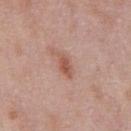| key | value |
|---|---|
| automated metrics | border irregularity of about 2.5 on a 0–10 scale; a nevus-likeness score of about 55/100 |
| lesion diameter | about 3 mm |
| tile lighting | white-light |
| location | the chest |
| subject | male, in their mid- to late 50s |
| image | 15 mm crop, total-body photography |